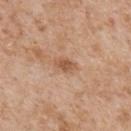Captured during whole-body skin photography for melanoma surveillance; the lesion was not biopsied.
On the chest.
A close-up tile cropped from a whole-body skin photograph, about 15 mm across.
This is a white-light tile.
A male patient roughly 65 years of age.
The recorded lesion diameter is about 2.5 mm.